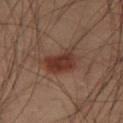Part of a total-body skin-imaging series; this lesion was reviewed on a skin check and was not flagged for biopsy.
A male patient aged approximately 55.
The lesion's longest dimension is about 4.5 mm.
Captured under cross-polarized illumination.
A 15 mm close-up extracted from a 3D total-body photography capture.
From the right thigh.
The lesion-visualizer software estimated a border-irregularity index near 3.5/10, a color-variation rating of about 3.5/10, and a peripheral color-asymmetry measure near 1. The analysis additionally found an automated nevus-likeness rating near 95 out of 100 and a lesion-detection confidence of about 100/100.